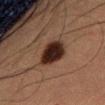Clinical impression:
No biopsy was performed on this lesion — it was imaged during a full skin examination and was not determined to be concerning.
Image and clinical context:
Measured at roughly 3.5 mm in maximum diameter. A roughly 15 mm field-of-view crop from a total-body skin photograph. Imaged with cross-polarized lighting. The lesion-visualizer software estimated a lesion color around L≈20 a*≈15 b*≈19 in CIELAB and a normalized lesion–skin contrast near 17. The lesion is on the front of the torso. A female patient, aged approximately 55.